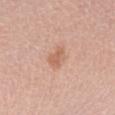{"biopsy_status": "not biopsied; imaged during a skin examination", "lesion_size": {"long_diameter_mm_approx": 2.5}, "lighting": "white-light", "patient": {"sex": "female", "age_approx": 65}, "image": {"source": "total-body photography crop", "field_of_view_mm": 15}, "site": "leg"}